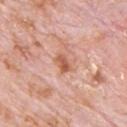Case summary:
- follow-up: imaged on a skin check; not biopsied
- tile lighting: white-light
- subject: male, roughly 80 years of age
- anatomic site: the front of the torso
- image-analysis metrics: an area of roughly 3.5 mm², an eccentricity of roughly 0.8, and a symmetry-axis asymmetry near 0.3
- diameter: ~2.5 mm (longest diameter)
- acquisition: ~15 mm crop, total-body skin-cancer survey